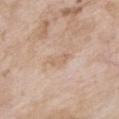workup=no biopsy performed (imaged during a skin exam) | subject=female, in their mid-70s | site=the front of the torso | lesion diameter=~3 mm (longest diameter) | tile lighting=white-light illumination | imaging modality=15 mm crop, total-body photography.